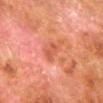follow-up: total-body-photography surveillance lesion; no biopsy | tile lighting: cross-polarized | acquisition: total-body-photography crop, ~15 mm field of view | patient: male, aged 78 to 82 | lesion size: about 3 mm | anatomic site: the right lower leg.